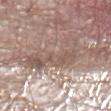Part of a total-body skin-imaging series; this lesion was reviewed on a skin check and was not flagged for biopsy.
Located on the left lower leg.
This is a white-light tile.
A lesion tile, about 15 mm wide, cut from a 3D total-body photograph.
The subject is a male approximately 80 years of age.
The lesion-visualizer software estimated an eccentricity of roughly 0.85 and two-axis asymmetry of about 0.65. The analysis additionally found a lesion color around L≈55 a*≈15 b*≈23 in CIELAB and about 8 CIELAB-L* units darker than the surrounding skin. The analysis additionally found a border-irregularity rating of about 9/10, a within-lesion color-variation index near 4.5/10, and radial color variation of about 1.5. The analysis additionally found a nevus-likeness score of about 0/100 and a lesion-detection confidence of about 5/100.
The lesion's longest dimension is about 7 mm.